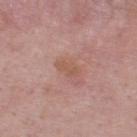Q: Was a biopsy performed?
A: total-body-photography surveillance lesion; no biopsy
Q: Where on the body is the lesion?
A: the upper back
Q: How large is the lesion?
A: ≈3 mm
Q: Illumination type?
A: white-light
Q: What kind of image is this?
A: 15 mm crop, total-body photography
Q: Patient demographics?
A: male, aged around 50